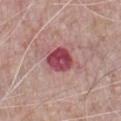{
  "biopsy_status": "not biopsied; imaged during a skin examination",
  "site": "front of the torso",
  "lesion_size": {
    "long_diameter_mm_approx": 3.0
  },
  "automated_metrics": {
    "area_mm2_approx": 8.5,
    "shape_asymmetry": 0.15,
    "lesion_detection_confidence_0_100": 100
  },
  "image": {
    "source": "total-body photography crop",
    "field_of_view_mm": 15
  },
  "patient": {
    "sex": "male",
    "age_approx": 70
  }
}